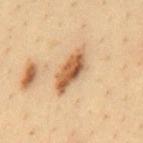{
  "patient": {
    "sex": "male",
    "age_approx": 35
  },
  "image": {
    "source": "total-body photography crop",
    "field_of_view_mm": 15
  },
  "site": "mid back",
  "lighting": "cross-polarized",
  "lesion_size": {
    "long_diameter_mm_approx": 5.5
  },
  "automated_metrics": {
    "cielab_L": 54,
    "cielab_a": 19,
    "cielab_b": 35,
    "vs_skin_darker_L": 15.0
  }
}